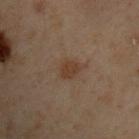Captured during whole-body skin photography for melanoma surveillance; the lesion was not biopsied. The tile uses cross-polarized illumination. About 2.5 mm across. Cropped from a whole-body photographic skin survey; the tile spans about 15 mm. A male subject aged around 50. From the upper back.